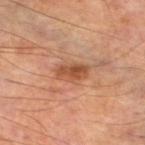* workup: imaged on a skin check; not biopsied
* acquisition: ~15 mm tile from a whole-body skin photo
* site: the left lower leg
* subject: male, roughly 55 years of age